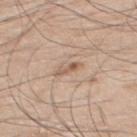The lesion was tiled from a total-body skin photograph and was not biopsied.
Located on the upper back.
A male subject roughly 70 years of age.
The tile uses white-light illumination.
The recorded lesion diameter is about 3 mm.
A 15 mm crop from a total-body photograph taken for skin-cancer surveillance.